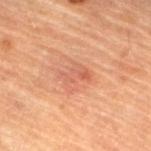Impression: The lesion was tiled from a total-body skin photograph and was not biopsied. Context: A male patient aged approximately 85 or older. A 15 mm close-up extracted from a 3D total-body photography capture. The lesion is on the left lower leg.